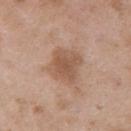- workup · total-body-photography surveillance lesion; no biopsy
- lesion size · ≈4 mm
- acquisition · total-body-photography crop, ~15 mm field of view
- lighting · white-light
- location · the right upper arm
- subject · male, aged around 50